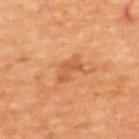Q: Is there a histopathology result?
A: no biopsy performed (imaged during a skin exam)
Q: What is the imaging modality?
A: total-body-photography crop, ~15 mm field of view
Q: How was the tile lit?
A: cross-polarized
Q: Lesion size?
A: ~3.5 mm (longest diameter)
Q: Patient demographics?
A: aged approximately 65
Q: Where on the body is the lesion?
A: the upper back
Q: Automated lesion metrics?
A: a mean CIELAB color near L≈57 a*≈27 b*≈42, about 8 CIELAB-L* units darker than the surrounding skin, and a lesion-to-skin contrast of about 5.5 (normalized; higher = more distinct)